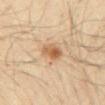| field | value |
|---|---|
| image-analysis metrics | a lesion color around L≈61 a*≈20 b*≈37 in CIELAB and roughly 12 lightness units darker than nearby skin; border irregularity of about 1.5 on a 0–10 scale, internal color variation of about 6 on a 0–10 scale, and radial color variation of about 2 |
| image | ~15 mm tile from a whole-body skin photo |
| subject | male, approximately 40 years of age |
| diameter | about 3.5 mm |
| illumination | cross-polarized |
| anatomic site | the abdomen |